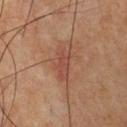biopsy status=imaged on a skin check; not biopsied | image source=~15 mm crop, total-body skin-cancer survey | lighting=cross-polarized | subject=male, about 65 years old | TBP lesion metrics=a lesion area of about 7.5 mm², an eccentricity of roughly 0.9, and a symmetry-axis asymmetry near 0.3; a mean CIELAB color near L≈45 a*≈22 b*≈28 and a normalized lesion–skin contrast near 5.5; an automated nevus-likeness rating near 5 out of 100 and a lesion-detection confidence of about 100/100 | anatomic site=the chest.